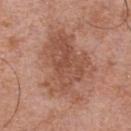| feature | finding |
|---|---|
| follow-up | total-body-photography surveillance lesion; no biopsy |
| imaging modality | ~15 mm tile from a whole-body skin photo |
| size | about 8.5 mm |
| subject | male, aged approximately 70 |
| lighting | white-light illumination |
| anatomic site | the chest |